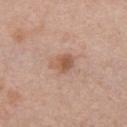| key | value |
|---|---|
| notes | no biopsy performed (imaged during a skin exam) |
| imaging modality | 15 mm crop, total-body photography |
| location | the chest |
| subject | female, roughly 40 years of age |
| TBP lesion metrics | a footprint of about 5 mm², a shape eccentricity near 0.65, and a shape-asymmetry score of about 0.25 (0 = symmetric); a mean CIELAB color near L≈56 a*≈21 b*≈30, roughly 10 lightness units darker than nearby skin, and a normalized border contrast of about 7 |
| illumination | white-light illumination |
| lesion diameter | ≈3 mm |